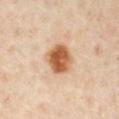Q: What kind of image is this?
A: 15 mm crop, total-body photography
Q: What is the lesion's diameter?
A: ~3.5 mm (longest diameter)
Q: How was the tile lit?
A: cross-polarized illumination
Q: Automated lesion metrics?
A: a lesion color around L≈58 a*≈23 b*≈38 in CIELAB and roughly 17 lightness units darker than nearby skin; a border-irregularity index near 1.5/10, a color-variation rating of about 6/10, and peripheral color asymmetry of about 2
Q: What is the anatomic site?
A: the chest
Q: Patient demographics?
A: male, about 65 years old